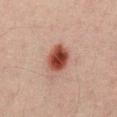<case>
<biopsy_status>not biopsied; imaged during a skin examination</biopsy_status>
<site>abdomen</site>
<image>
  <source>total-body photography crop</source>
  <field_of_view_mm>15</field_of_view_mm>
</image>
<lighting>cross-polarized</lighting>
<patient>
  <sex>male</sex>
  <age_approx>30</age_approx>
</patient>
</case>